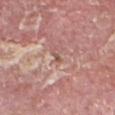patient=male, approximately 65 years of age; anatomic site=the head or neck; lighting=white-light; imaging modality=~15 mm tile from a whole-body skin photo; lesion size=~1.5 mm (longest diameter); biopsy diagnosis=a squamous cell carcinoma in situ.